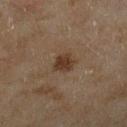- automated metrics · an area of roughly 5 mm², an outline eccentricity of about 0.55 (0 = round, 1 = elongated), and two-axis asymmetry of about 0.2; a nevus-likeness score of about 95/100
- acquisition · ~15 mm tile from a whole-body skin photo
- lighting · cross-polarized
- patient · female, aged 58 to 62
- size · ≈2.5 mm
- site · the leg